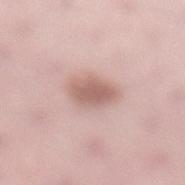workup: no biopsy performed (imaged during a skin exam); illumination: white-light; patient: female, in their mid- to late 20s; anatomic site: the left lower leg; image source: ~15 mm tile from a whole-body skin photo.